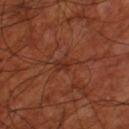Impression: Imaged during a routine full-body skin examination; the lesion was not biopsied and no histopathology is available. Context: A lesion tile, about 15 mm wide, cut from a 3D total-body photograph. The lesion is located on the left thigh. Imaged with cross-polarized lighting. The total-body-photography lesion software estimated an area of roughly 4.5 mm², an outline eccentricity of about 0.75 (0 = round, 1 = elongated), and a symmetry-axis asymmetry near 0.3. And it measured a nevus-likeness score of about 0/100. A male patient roughly 70 years of age.